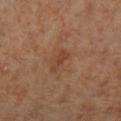biopsy_status: not biopsied; imaged during a skin examination
lesion_size:
  long_diameter_mm_approx: 3.0
image:
  source: total-body photography crop
  field_of_view_mm: 15
patient:
  sex: female
  age_approx: 70
automated_metrics:
  cielab_L: 43
  cielab_a: 22
  cielab_b: 32
  vs_skin_darker_L: 7.0
  vs_skin_contrast_norm: 6.0
  color_variation_0_10: 0.0
  peripheral_color_asymmetry: 0.0
  nevus_likeness_0_100: 0
  lesion_detection_confidence_0_100: 100
site: left lower leg
lighting: cross-polarized